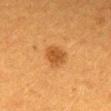{"biopsy_status": "not biopsied; imaged during a skin examination", "lesion_size": {"long_diameter_mm_approx": 3.0}, "site": "back", "automated_metrics": {"nevus_likeness_0_100": 100, "lesion_detection_confidence_0_100": 100}, "image": {"source": "total-body photography crop", "field_of_view_mm": 15}, "patient": {"sex": "female", "age_approx": 40}}